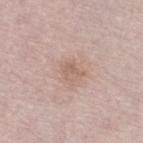Captured during whole-body skin photography for melanoma surveillance; the lesion was not biopsied.
A male patient, in their mid-60s.
Measured at roughly 2.5 mm in maximum diameter.
This is a white-light tile.
A roughly 15 mm field-of-view crop from a total-body skin photograph.
The lesion-visualizer software estimated border irregularity of about 3 on a 0–10 scale and radial color variation of about 1.
On the leg.